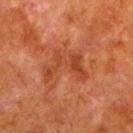Impression: The lesion was tiled from a total-body skin photograph and was not biopsied. Acquisition and patient details: A male subject aged 78 to 82. A roughly 15 mm field-of-view crop from a total-body skin photograph. The total-body-photography lesion software estimated a border-irregularity rating of about 10/10 and a peripheral color-asymmetry measure near 0.5. And it measured a nevus-likeness score of about 0/100 and a lesion-detection confidence of about 100/100. The lesion's longest dimension is about 5.5 mm. Located on the left lower leg. Captured under cross-polarized illumination.